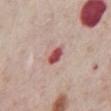Imaged during a routine full-body skin examination; the lesion was not biopsied and no histopathology is available. About 2.5 mm across. Automated image analysis of the tile measured a footprint of about 4 mm² and an eccentricity of roughly 0.75. The analysis additionally found border irregularity of about 2 on a 0–10 scale, a within-lesion color-variation index near 3/10, and a peripheral color-asymmetry measure near 1. The lesion is on the abdomen. A male subject, aged 73 to 77. A 15 mm close-up extracted from a 3D total-body photography capture.